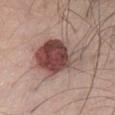{"biopsy_status": "not biopsied; imaged during a skin examination", "lighting": "white-light", "image": {"source": "total-body photography crop", "field_of_view_mm": 15}, "automated_metrics": {"area_mm2_approx": 24.0, "eccentricity": 0.5, "shape_asymmetry": 0.25, "cielab_L": 47, "cielab_a": 20, "cielab_b": 22, "vs_skin_darker_L": 16.0, "vs_skin_contrast_norm": 11.0, "border_irregularity_0_10": 3.0, "peripheral_color_asymmetry": 3.0}, "lesion_size": {"long_diameter_mm_approx": 6.0}, "site": "front of the torso", "patient": {"sex": "male", "age_approx": 40}}